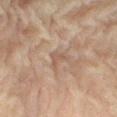workup: catalogued during a skin exam; not biopsied
subject: female, approximately 80 years of age
anatomic site: the right thigh
image: ~15 mm tile from a whole-body skin photo